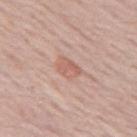| key | value |
|---|---|
| biopsy status | no biopsy performed (imaged during a skin exam) |
| tile lighting | white-light |
| image source | total-body-photography crop, ~15 mm field of view |
| patient | female, aged 58 to 62 |
| site | the left thigh |
| size | ~2.5 mm (longest diameter) |